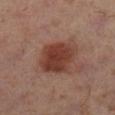<tbp_lesion>
  <biopsy_status>not biopsied; imaged during a skin examination</biopsy_status>
  <site>left lower leg</site>
  <image>
    <source>total-body photography crop</source>
    <field_of_view_mm>15</field_of_view_mm>
  </image>
  <patient>
    <sex>female</sex>
    <age_approx>40</age_approx>
  </patient>
  <lesion_size>
    <long_diameter_mm_approx>5.0</long_diameter_mm_approx>
  </lesion_size>
  <lighting>cross-polarized</lighting>
</tbp_lesion>